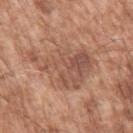Captured during whole-body skin photography for melanoma surveillance; the lesion was not biopsied.
From the left upper arm.
The lesion's longest dimension is about 7 mm.
Imaged with white-light lighting.
A male subject aged around 65.
A 15 mm close-up extracted from a 3D total-body photography capture.